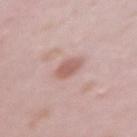Clinical impression: Imaged during a routine full-body skin examination; the lesion was not biopsied and no histopathology is available. Image and clinical context: Approximately 3 mm at its widest. A female subject, aged 13–17. A 15 mm crop from a total-body photograph taken for skin-cancer surveillance. Captured under white-light illumination. On the left upper arm.